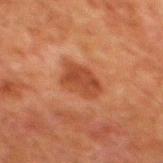Notes:
* workup: no biopsy performed (imaged during a skin exam)
* lesion size: about 4 mm
* imaging modality: 15 mm crop, total-body photography
* location: the mid back
* automated lesion analysis: a border-irregularity rating of about 2/10, internal color variation of about 2.5 on a 0–10 scale, and peripheral color asymmetry of about 1; an automated nevus-likeness rating near 60 out of 100
* subject: male, approximately 80 years of age